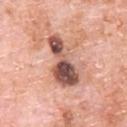A region of skin cropped from a whole-body photographic capture, roughly 15 mm wide.
On the upper back.
Longest diameter approximately 6.5 mm.
The subject is a male aged 78–82.